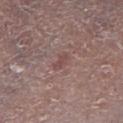{
  "biopsy_status": "not biopsied; imaged during a skin examination",
  "site": "right lower leg",
  "patient": {
    "sex": "male",
    "age_approx": 80
  },
  "lesion_size": {
    "long_diameter_mm_approx": 2.5
  },
  "automated_metrics": {
    "eccentricity": 0.85,
    "shape_asymmetry": 0.35,
    "nevus_likeness_0_100": 0,
    "lesion_detection_confidence_0_100": 100
  },
  "image": {
    "source": "total-body photography crop",
    "field_of_view_mm": 15
  }
}